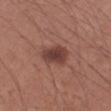<record>
<biopsy_status>not biopsied; imaged during a skin examination</biopsy_status>
<image>
  <source>total-body photography crop</source>
  <field_of_view_mm>15</field_of_view_mm>
</image>
<patient>
  <sex>male</sex>
  <age_approx>55</age_approx>
</patient>
<site>right upper arm</site>
</record>